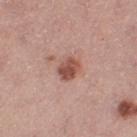{"biopsy_status": "not biopsied; imaged during a skin examination", "site": "left thigh", "image": {"source": "total-body photography crop", "field_of_view_mm": 15}, "lighting": "white-light", "patient": {"sex": "female", "age_approx": 40}}